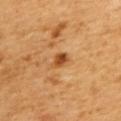Clinical impression:
Recorded during total-body skin imaging; not selected for excision or biopsy.
Context:
This is a cross-polarized tile. The recorded lesion diameter is about 2 mm. The subject is a female aged 53–57. On the back. A roughly 15 mm field-of-view crop from a total-body skin photograph.